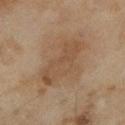Clinical summary: The patient is a female aged 58 to 62. A region of skin cropped from a whole-body photographic capture, roughly 15 mm wide. About 7 mm across. Located on the left lower leg.